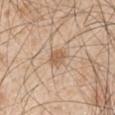The lesion was tiled from a total-body skin photograph and was not biopsied.
The recorded lesion diameter is about 2.5 mm.
From the right upper arm.
A region of skin cropped from a whole-body photographic capture, roughly 15 mm wide.
A male subject in their 50s.
Automated image analysis of the tile measured a border-irregularity index near 2.5/10 and a within-lesion color-variation index near 1.5/10. And it measured an automated nevus-likeness rating near 85 out of 100.
Captured under white-light illumination.